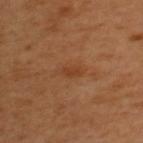Assessment:
The lesion was photographed on a routine skin check and not biopsied; there is no pathology result.
Context:
The total-body-photography lesion software estimated an area of roughly 2.5 mm², an eccentricity of roughly 0.9, and a symmetry-axis asymmetry near 0.25. The analysis additionally found a mean CIELAB color near L≈40 a*≈23 b*≈35, about 6 CIELAB-L* units darker than the surrounding skin, and a lesion-to-skin contrast of about 5.5 (normalized; higher = more distinct). And it measured a nevus-likeness score of about 5/100 and a lesion-detection confidence of about 100/100. A male subject aged approximately 50. About 2.5 mm across. A 15 mm crop from a total-body photograph taken for skin-cancer surveillance. Located on the upper back. Captured under cross-polarized illumination.